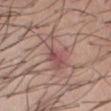The lesion was tiled from a total-body skin photograph and was not biopsied. From the abdomen. The tile uses white-light illumination. The subject is a male roughly 35 years of age. Longest diameter approximately 5 mm. A lesion tile, about 15 mm wide, cut from a 3D total-body photograph. Automated image analysis of the tile measured roughly 8 lightness units darker than nearby skin and a normalized border contrast of about 6. And it measured a border-irregularity rating of about 6/10 and a peripheral color-asymmetry measure near 1. It also reported a classifier nevus-likeness of about 0/100 and a detector confidence of about 95 out of 100 that the crop contains a lesion.